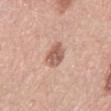Case summary:
• notes — catalogued during a skin exam; not biopsied
• image source — 15 mm crop, total-body photography
• lighting — white-light
• automated lesion analysis — about 12 CIELAB-L* units darker than the surrounding skin and a normalized lesion–skin contrast near 8; a border-irregularity index near 2/10 and peripheral color asymmetry of about 1.5; an automated nevus-likeness rating near 30 out of 100 and a lesion-detection confidence of about 100/100
• body site — the left lower leg
• patient — female, in their mid- to late 60s